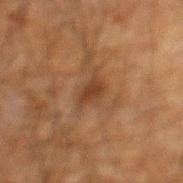  biopsy_status: not biopsied; imaged during a skin examination
  site: mid back
  patient:
    sex: male
    age_approx: 60
  automated_metrics:
    area_mm2_approx: 4.0
    eccentricity: 0.8
    shape_asymmetry: 0.3
    vs_skin_darker_L: 7.0
    vs_skin_contrast_norm: 7.5
    nevus_likeness_0_100: 45
  lighting: cross-polarized
  image:
    source: total-body photography crop
    field_of_view_mm: 15